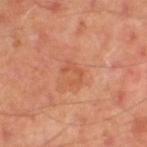notes = imaged on a skin check; not biopsied | patient = male, about 50 years old | imaging modality = total-body-photography crop, ~15 mm field of view | automated lesion analysis = border irregularity of about 8.5 on a 0–10 scale, a color-variation rating of about 0/10, and a peripheral color-asymmetry measure near 0 | anatomic site = the left leg | lesion diameter = ≈2.5 mm.